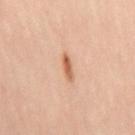{"site": "leg", "image": {"source": "total-body photography crop", "field_of_view_mm": 15}, "patient": {"sex": "female", "age_approx": 40}}